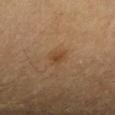Recorded during total-body skin imaging; not selected for excision or biopsy.
Captured under cross-polarized illumination.
A close-up tile cropped from a whole-body skin photograph, about 15 mm across.
Located on the right forearm.
The subject is a female in their 50s.
Longest diameter approximately 2.5 mm.